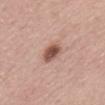Case summary:
• biopsy status: catalogued during a skin exam; not biopsied
• lighting: white-light
• subject: male, aged approximately 60
• image source: total-body-photography crop, ~15 mm field of view
• location: the abdomen
• automated lesion analysis: a lesion color around L≈53 a*≈21 b*≈27 in CIELAB, about 15 CIELAB-L* units darker than the surrounding skin, and a normalized lesion–skin contrast near 10; a classifier nevus-likeness of about 95/100 and a lesion-detection confidence of about 100/100
• size: ~3 mm (longest diameter)